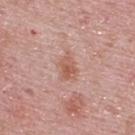Background: A male patient aged 48 to 52. Approximately 3.5 mm at its widest. Automated tile analysis of the lesion measured internal color variation of about 3 on a 0–10 scale and a peripheral color-asymmetry measure near 1. And it measured a detector confidence of about 100 out of 100 that the crop contains a lesion. On the upper back. Cropped from a total-body skin-imaging series; the visible field is about 15 mm.